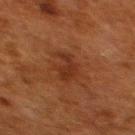  biopsy_status: not biopsied; imaged during a skin examination
  site: upper back
  patient:
    sex: female
    age_approx: 50
  image:
    source: total-body photography crop
    field_of_view_mm: 15
  lesion_size:
    long_diameter_mm_approx: 3.5